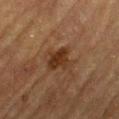Part of a total-body skin-imaging series; this lesion was reviewed on a skin check and was not flagged for biopsy.
A male subject, in their mid-80s or older.
Imaged with cross-polarized lighting.
The total-body-photography lesion software estimated a lesion–skin lightness drop of about 8 and a normalized lesion–skin contrast near 8.5. The analysis additionally found an automated nevus-likeness rating near 70 out of 100 and a lesion-detection confidence of about 100/100.
Longest diameter approximately 3.5 mm.
A 15 mm close-up extracted from a 3D total-body photography capture.
Located on the left thigh.